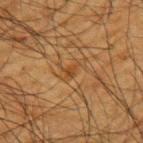follow-up: no biopsy performed (imaged during a skin exam)
subject: male, approximately 65 years of age
tile lighting: cross-polarized
imaging modality: ~15 mm crop, total-body skin-cancer survey
lesion diameter: about 2.5 mm
location: the left upper arm